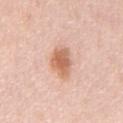Findings:
* follow-up — total-body-photography surveillance lesion; no biopsy
* patient — male, in their 50s
* imaging modality — ~15 mm tile from a whole-body skin photo
* body site — the chest